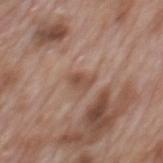notes: catalogued during a skin exam; not biopsied | illumination: white-light illumination | automated lesion analysis: an eccentricity of roughly 0.7 and a shape-asymmetry score of about 0.3 (0 = symmetric) | location: the mid back | imaging modality: ~15 mm tile from a whole-body skin photo | subject: male, aged 68–72.